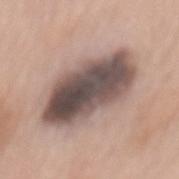{"biopsy_status": "not biopsied; imaged during a skin examination", "lighting": "white-light", "patient": {"sex": "female", "age_approx": 60}, "image": {"source": "total-body photography crop", "field_of_view_mm": 15}, "automated_metrics": {"area_mm2_approx": 34.0, "eccentricity": 0.85, "shape_asymmetry": 0.2, "cielab_L": 49, "cielab_a": 14, "cielab_b": 19, "vs_skin_contrast_norm": 13.0, "border_irregularity_0_10": 2.5, "color_variation_0_10": 9.5, "peripheral_color_asymmetry": 3.5, "nevus_likeness_0_100": 0, "lesion_detection_confidence_0_100": 80}, "lesion_size": {"long_diameter_mm_approx": 9.5}, "site": "back"}